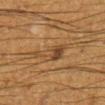<lesion>
<biopsy_status>not biopsied; imaged during a skin examination</biopsy_status>
<lesion_size>
  <long_diameter_mm_approx>5.0</long_diameter_mm_approx>
</lesion_size>
<image>
  <source>total-body photography crop</source>
  <field_of_view_mm>15</field_of_view_mm>
</image>
<patient>
  <sex>male</sex>
  <age_approx>60</age_approx>
</patient>
<site>right lower leg</site>
</lesion>